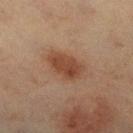The lesion was tiled from a total-body skin photograph and was not biopsied.
From the right lower leg.
A lesion tile, about 15 mm wide, cut from a 3D total-body photograph.
Approximately 4.5 mm at its widest.
Imaged with cross-polarized lighting.
Automated image analysis of the tile measured a lesion color around L≈38 a*≈19 b*≈28 in CIELAB and about 9 CIELAB-L* units darker than the surrounding skin. The analysis additionally found a classifier nevus-likeness of about 100/100 and a lesion-detection confidence of about 100/100.
A female subject, about 55 years old.